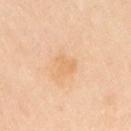No biopsy was performed on this lesion — it was imaged during a full skin examination and was not determined to be concerning. A female subject about 65 years old. Located on the arm. Automated tile analysis of the lesion measured a footprint of about 4.5 mm² and an eccentricity of roughly 0.6. And it measured a classifier nevus-likeness of about 0/100 and a detector confidence of about 100 out of 100 that the crop contains a lesion. A lesion tile, about 15 mm wide, cut from a 3D total-body photograph. Captured under white-light illumination.